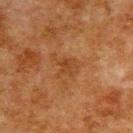Assessment:
The lesion was photographed on a routine skin check and not biopsied; there is no pathology result.
Clinical summary:
On the back. A lesion tile, about 15 mm wide, cut from a 3D total-body photograph. A male patient approximately 80 years of age.